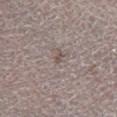workup: catalogued during a skin exam; not biopsied | body site: the left lower leg | lesion diameter: ≈1.5 mm | lighting: white-light | acquisition: total-body-photography crop, ~15 mm field of view | subject: male, aged 53–57.